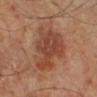biopsy status: catalogued during a skin exam; not biopsied
image source: ~15 mm tile from a whole-body skin photo
location: the right lower leg
patient: male, about 70 years old